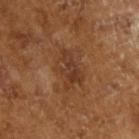Imaged during a routine full-body skin examination; the lesion was not biopsied and no histopathology is available.
A lesion tile, about 15 mm wide, cut from a 3D total-body photograph.
The tile uses cross-polarized illumination.
A male subject aged approximately 65.
Approximately 4 mm at its widest.